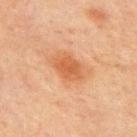biopsy status: no biopsy performed (imaged during a skin exam) | tile lighting: cross-polarized | automated lesion analysis: an area of roughly 7 mm², an outline eccentricity of about 0.75 (0 = round, 1 = elongated), and two-axis asymmetry of about 0.2; a border-irregularity rating of about 2/10, a within-lesion color-variation index near 2/10, and peripheral color asymmetry of about 0.5; a nevus-likeness score of about 90/100 and a detector confidence of about 100 out of 100 that the crop contains a lesion | anatomic site: the chest | acquisition: ~15 mm tile from a whole-body skin photo | patient: male, aged 68 to 72.